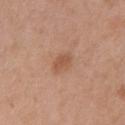Captured during whole-body skin photography for melanoma surveillance; the lesion was not biopsied. The subject is a female aged approximately 40. Located on the chest. Approximately 3 mm at its widest. The total-body-photography lesion software estimated a lesion-to-skin contrast of about 6 (normalized; higher = more distinct). The analysis additionally found an automated nevus-likeness rating near 35 out of 100. A region of skin cropped from a whole-body photographic capture, roughly 15 mm wide.